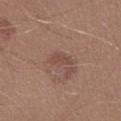Assessment: Imaged during a routine full-body skin examination; the lesion was not biopsied and no histopathology is available.